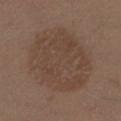Q: What did automated image analysis measure?
A: an area of roughly 50 mm², an eccentricity of roughly 0.55, and a symmetry-axis asymmetry near 0.1; a lesion color around L≈42 a*≈15 b*≈24 in CIELAB, about 6 CIELAB-L* units darker than the surrounding skin, and a lesion-to-skin contrast of about 5 (normalized; higher = more distinct); a border-irregularity index near 1.5/10, a color-variation rating of about 3/10, and peripheral color asymmetry of about 1; an automated nevus-likeness rating near 10 out of 100 and lesion-presence confidence of about 100/100
Q: Lesion size?
A: ≈9 mm
Q: Who is the patient?
A: female, approximately 40 years of age
Q: What is the imaging modality?
A: ~15 mm crop, total-body skin-cancer survey
Q: Lesion location?
A: the leg
Q: What lighting was used for the tile?
A: white-light illumination